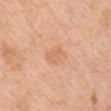follow-up = imaged on a skin check; not biopsied
lighting = white-light illumination
subject = female, aged 43 to 47
lesion size = ~3 mm (longest diameter)
imaging modality = ~15 mm crop, total-body skin-cancer survey
anatomic site = the arm
image-analysis metrics = a lesion area of about 5 mm², a shape eccentricity near 0.65, and a symmetry-axis asymmetry near 0.2; an automated nevus-likeness rating near 0 out of 100 and a lesion-detection confidence of about 100/100